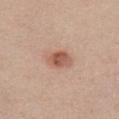Clinical impression: No biopsy was performed on this lesion — it was imaged during a full skin examination and was not determined to be concerning. Acquisition and patient details: The lesion's longest dimension is about 3 mm. The subject is a female aged 53–57. A 15 mm close-up extracted from a 3D total-body photography capture. Captured under white-light illumination. On the chest. An algorithmic analysis of the crop reported a lesion color around L≈56 a*≈22 b*≈29 in CIELAB, a lesion–skin lightness drop of about 12, and a normalized border contrast of about 8. The software also gave border irregularity of about 2 on a 0–10 scale and internal color variation of about 4.5 on a 0–10 scale.